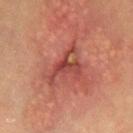{"biopsy_status": "not biopsied; imaged during a skin examination", "site": "chest", "lesion_size": {"long_diameter_mm_approx": 5.0}, "automated_metrics": {"area_mm2_approx": 7.5, "eccentricity": 0.7, "shape_asymmetry": 0.7, "cielab_L": 45, "cielab_a": 32, "cielab_b": 28, "vs_skin_darker_L": 10.0, "vs_skin_contrast_norm": 7.5, "border_irregularity_0_10": 10.0, "color_variation_0_10": 5.5, "peripheral_color_asymmetry": 2.0}, "patient": {"sex": "female", "age_approx": 35}, "image": {"source": "total-body photography crop", "field_of_view_mm": 15}}